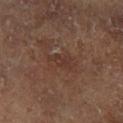biopsy status — no biopsy performed (imaged during a skin exam) | location — the left lower leg | imaging modality — ~15 mm crop, total-body skin-cancer survey | subject — male, approximately 65 years of age.